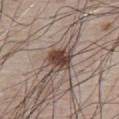This lesion was catalogued during total-body skin photography and was not selected for biopsy. Located on the front of the torso. A male subject, aged 78 to 82. This image is a 15 mm lesion crop taken from a total-body photograph. The tile uses white-light illumination.